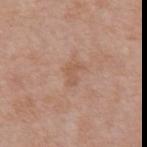The lesion was photographed on a routine skin check and not biopsied; there is no pathology result. The lesion is on the abdomen. About 2.5 mm across. A 15 mm close-up tile from a total-body photography series done for melanoma screening. Automated image analysis of the tile measured a mean CIELAB color near L≈56 a*≈20 b*≈31 and about 6 CIELAB-L* units darker than the surrounding skin. The analysis additionally found border irregularity of about 5 on a 0–10 scale. The subject is a male aged approximately 70. Imaged with white-light lighting.